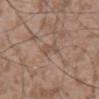<lesion>
<biopsy_status>not biopsied; imaged during a skin examination</biopsy_status>
<automated_metrics>
  <area_mm2_approx>2.5</area_mm2_approx>
  <eccentricity>0.9</eccentricity>
  <shape_asymmetry>0.35</shape_asymmetry>
  <cielab_L>51</cielab_L>
  <cielab_a>16</cielab_a>
  <cielab_b>27</cielab_b>
  <vs_skin_darker_L>6.0</vs_skin_darker_L>
  <vs_skin_contrast_norm>5.0</vs_skin_contrast_norm>
  <peripheral_color_asymmetry>1.0</peripheral_color_asymmetry>
  <lesion_detection_confidence_0_100>70</lesion_detection_confidence_0_100>
</automated_metrics>
<lesion_size>
  <long_diameter_mm_approx>2.5</long_diameter_mm_approx>
</lesion_size>
<lighting>white-light</lighting>
<image>
  <source>total-body photography crop</source>
  <field_of_view_mm>15</field_of_view_mm>
</image>
<site>abdomen</site>
<patient>
  <sex>male</sex>
  <age_approx>55</age_approx>
</patient>
</lesion>